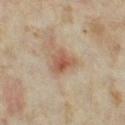The lesion was photographed on a routine skin check and not biopsied; there is no pathology result. A close-up tile cropped from a whole-body skin photograph, about 15 mm across. Located on the right thigh. Automated image analysis of the tile measured a lesion area of about 6 mm², a shape eccentricity near 0.6, and a symmetry-axis asymmetry near 0.4. The patient is a female roughly 35 years of age. The lesion's longest dimension is about 3 mm.